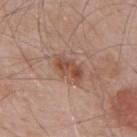The lesion was tiled from a total-body skin photograph and was not biopsied. About 4.5 mm across. Automated tile analysis of the lesion measured a lesion color around L≈50 a*≈21 b*≈29 in CIELAB, roughly 10 lightness units darker than nearby skin, and a normalized lesion–skin contrast near 7.5. The software also gave internal color variation of about 4.5 on a 0–10 scale and a peripheral color-asymmetry measure near 1.5. A close-up tile cropped from a whole-body skin photograph, about 15 mm across. Captured under white-light illumination. Located on the mid back. A male subject in their mid- to late 50s.